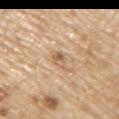Assessment:
Captured during whole-body skin photography for melanoma surveillance; the lesion was not biopsied.
Background:
A male patient roughly 70 years of age. Cropped from a whole-body photographic skin survey; the tile spans about 15 mm. Measured at roughly 2.5 mm in maximum diameter. From the right upper arm. Captured under white-light illumination.